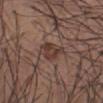Captured during whole-body skin photography for melanoma surveillance; the lesion was not biopsied. Located on the right forearm. A male patient, in their 50s. A roughly 15 mm field-of-view crop from a total-body skin photograph. Imaged with white-light lighting. Longest diameter approximately 3 mm.